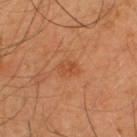Case summary:
– workup — no biopsy performed (imaged during a skin exam)
– patient — male, aged approximately 45
– image source — ~15 mm tile from a whole-body skin photo
– diameter — about 2.5 mm
– automated lesion analysis — a nevus-likeness score of about 25/100 and a lesion-detection confidence of about 100/100
– body site — the back
– lighting — cross-polarized